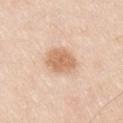The lesion was photographed on a routine skin check and not biopsied; there is no pathology result. A male subject about 35 years old. The tile uses white-light illumination. Longest diameter approximately 4 mm. From the right upper arm. Automated tile analysis of the lesion measured an area of roughly 9.5 mm², an eccentricity of roughly 0.5, and two-axis asymmetry of about 0.15. The software also gave an average lesion color of about L≈67 a*≈20 b*≈34 (CIELAB) and about 12 CIELAB-L* units darker than the surrounding skin. It also reported a within-lesion color-variation index near 2.5/10. It also reported a classifier nevus-likeness of about 65/100 and a lesion-detection confidence of about 100/100. This image is a 15 mm lesion crop taken from a total-body photograph.